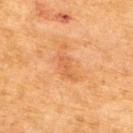Case summary:
• biopsy status — no biopsy performed (imaged during a skin exam)
• subject — male, aged approximately 65
• illumination — cross-polarized
• imaging modality — ~15 mm crop, total-body skin-cancer survey
• automated metrics — a border-irregularity index near 3.5/10, a color-variation rating of about 3/10, and peripheral color asymmetry of about 1; a nevus-likeness score of about 0/100 and lesion-presence confidence of about 100/100